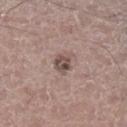The lesion was tiled from a total-body skin photograph and was not biopsied. Cropped from a total-body skin-imaging series; the visible field is about 15 mm. Approximately 3 mm at its widest. Located on the right lower leg. The tile uses white-light illumination. A male subject, aged 43 to 47. The total-body-photography lesion software estimated a lesion area of about 5 mm², a shape eccentricity near 0.7, and a shape-asymmetry score of about 0.3 (0 = symmetric). The analysis additionally found a nevus-likeness score of about 15/100.